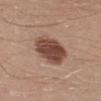| key | value |
|---|---|
| automated lesion analysis | a lesion–skin lightness drop of about 15 and a lesion-to-skin contrast of about 11 (normalized; higher = more distinct) |
| anatomic site | the right lower leg |
| tile lighting | white-light |
| subject | male, aged 28 to 32 |
| size | ≈5 mm |
| imaging modality | ~15 mm crop, total-body skin-cancer survey |